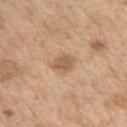  biopsy_status: not biopsied; imaged during a skin examination
  patient:
    sex: male
    age_approx: 70
  automated_metrics:
    cielab_L: 58
    cielab_a: 19
    cielab_b: 33
    vs_skin_darker_L: 10.0
    vs_skin_contrast_norm: 6.5
    nevus_likeness_0_100: 35
    lesion_detection_confidence_0_100: 100
  image:
    source: total-body photography crop
    field_of_view_mm: 15
  site: left upper arm
  lesion_size:
    long_diameter_mm_approx: 3.0